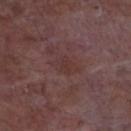{
  "biopsy_status": "not biopsied; imaged during a skin examination",
  "image": {
    "source": "total-body photography crop",
    "field_of_view_mm": 15
  },
  "site": "chest",
  "automated_metrics": {
    "area_mm2_approx": 6.0,
    "eccentricity": 0.8,
    "shape_asymmetry": 0.3
  },
  "lighting": "white-light",
  "patient": {
    "sex": "male",
    "age_approx": 65
  }
}